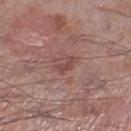This lesion was catalogued during total-body skin photography and was not selected for biopsy.
A close-up tile cropped from a whole-body skin photograph, about 15 mm across.
From the right lower leg.
A male subject approximately 70 years of age.